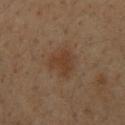This is a cross-polarized tile.
About 4 mm across.
A roughly 15 mm field-of-view crop from a total-body skin photograph.
A male patient aged around 35.
The lesion is located on the upper back.